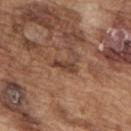Captured during whole-body skin photography for melanoma surveillance; the lesion was not biopsied. On the upper back. Measured at roughly 3 mm in maximum diameter. A 15 mm close-up extracted from a 3D total-body photography capture. The subject is a male in their mid-70s. This is a white-light tile.